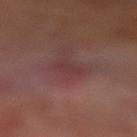follow-up: catalogued during a skin exam; not biopsied | image source: total-body-photography crop, ~15 mm field of view | automated metrics: a lesion area of about 4 mm², an eccentricity of roughly 0.7, and a shape-asymmetry score of about 0.3 (0 = symmetric); a border-irregularity rating of about 3/10, internal color variation of about 1 on a 0–10 scale, and peripheral color asymmetry of about 0.5; a nevus-likeness score of about 0/100 and a lesion-detection confidence of about 85/100 | patient: male, approximately 70 years of age | lighting: cross-polarized | lesion size: ~2.5 mm (longest diameter) | anatomic site: the left lower leg.